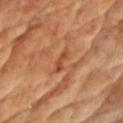| key | value |
|---|---|
| workup | no biopsy performed (imaged during a skin exam) |
| acquisition | total-body-photography crop, ~15 mm field of view |
| lighting | cross-polarized |
| anatomic site | the front of the torso |
| automated lesion analysis | a lesion color around L≈47 a*≈26 b*≈38 in CIELAB, a lesion–skin lightness drop of about 9, and a lesion-to-skin contrast of about 7 (normalized; higher = more distinct); border irregularity of about 4 on a 0–10 scale and a within-lesion color-variation index near 0/10 |
| subject | male, approximately 60 years of age |